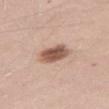Clinical summary: On the right thigh. A female subject, aged 28 to 32. A 15 mm crop from a total-body photograph taken for skin-cancer surveillance.